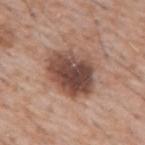Q: Is there a histopathology result?
A: imaged on a skin check; not biopsied
Q: What is the lesion's diameter?
A: ≈6.5 mm
Q: What is the imaging modality?
A: 15 mm crop, total-body photography
Q: Automated lesion metrics?
A: an average lesion color of about L≈48 a*≈19 b*≈25 (CIELAB), roughly 15 lightness units darker than nearby skin, and a normalized lesion–skin contrast near 10.5; a detector confidence of about 100 out of 100 that the crop contains a lesion
Q: What lighting was used for the tile?
A: white-light
Q: What are the patient's age and sex?
A: male, roughly 60 years of age
Q: Where on the body is the lesion?
A: the chest